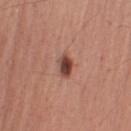workup: catalogued during a skin exam; not biopsied | imaging modality: ~15 mm crop, total-body skin-cancer survey | subject: male, aged 58–62 | anatomic site: the right upper arm.